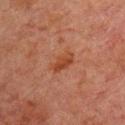follow-up = no biopsy performed (imaged during a skin exam); patient = male, roughly 60 years of age; automated metrics = a border-irregularity index near 3/10, internal color variation of about 2.5 on a 0–10 scale, and radial color variation of about 1; body site = the chest; diameter = about 3.5 mm; tile lighting = cross-polarized; image = ~15 mm tile from a whole-body skin photo.